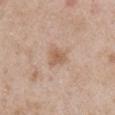workup: imaged on a skin check; not biopsied | illumination: white-light illumination | imaging modality: 15 mm crop, total-body photography | diameter: about 2.5 mm | patient: male, aged 48 to 52 | automated lesion analysis: a mean CIELAB color near L≈59 a*≈18 b*≈31, a lesion–skin lightness drop of about 9, and a lesion-to-skin contrast of about 6.5 (normalized; higher = more distinct) | location: the right upper arm.